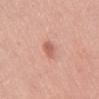Captured during whole-body skin photography for melanoma surveillance; the lesion was not biopsied. Located on the mid back. Imaged with white-light lighting. This image is a 15 mm lesion crop taken from a total-body photograph. A female subject, aged 53 to 57.